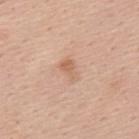The lesion is on the upper back.
A male subject aged 53–57.
This image is a 15 mm lesion crop taken from a total-body photograph.